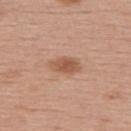The lesion was tiled from a total-body skin photograph and was not biopsied. Imaged with white-light lighting. This image is a 15 mm lesion crop taken from a total-body photograph. A female subject, about 55 years old. Longest diameter approximately 3.5 mm. The lesion is located on the upper back.